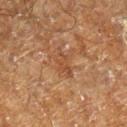{"biopsy_status": "not biopsied; imaged during a skin examination"}